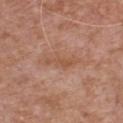  biopsy_status: not biopsied; imaged during a skin examination
  image:
    source: total-body photography crop
    field_of_view_mm: 15
  lighting: white-light
  site: chest
  lesion_size:
    long_diameter_mm_approx: 4.0
  patient:
    sex: male
    age_approx: 75